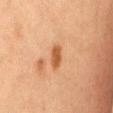The lesion was tiled from a total-body skin photograph and was not biopsied.